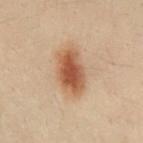Q: Was a biopsy performed?
A: total-body-photography surveillance lesion; no biopsy
Q: What is the anatomic site?
A: the front of the torso
Q: Patient demographics?
A: male, aged approximately 30
Q: What is the imaging modality?
A: ~15 mm tile from a whole-body skin photo
Q: How was the tile lit?
A: cross-polarized illumination
Q: What is the lesion's diameter?
A: ~5.5 mm (longest diameter)
Q: Automated lesion metrics?
A: a shape eccentricity near 0.8 and two-axis asymmetry of about 0.15; roughly 11 lightness units darker than nearby skin and a normalized border contrast of about 9; a border-irregularity index near 1.5/10 and peripheral color asymmetry of about 1; an automated nevus-likeness rating near 100 out of 100 and a detector confidence of about 100 out of 100 that the crop contains a lesion